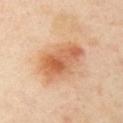- biopsy status · catalogued during a skin exam; not biopsied
- subject · female, aged 38–42
- body site · the left upper arm
- imaging modality · 15 mm crop, total-body photography
- lesion diameter · ~5.5 mm (longest diameter)